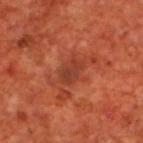<lesion>
  <biopsy_status>not biopsied; imaged during a skin examination</biopsy_status>
  <lighting>cross-polarized</lighting>
  <image>
    <source>total-body photography crop</source>
    <field_of_view_mm>15</field_of_view_mm>
  </image>
  <automated_metrics>
    <nevus_likeness_0_100>0</nevus_likeness_0_100>
    <lesion_detection_confidence_0_100>100</lesion_detection_confidence_0_100>
  </automated_metrics>
  <patient>
    <sex>male</sex>
    <age_approx>70</age_approx>
  </patient>
  <site>upper back</site>
  <lesion_size>
    <long_diameter_mm_approx>3.0</long_diameter_mm_approx>
  </lesion_size>
</lesion>